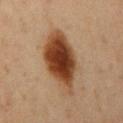Findings:
– follow-up — total-body-photography surveillance lesion; no biopsy
– imaging modality — ~15 mm crop, total-body skin-cancer survey
– site — the arm
– patient — female, aged 43 to 47